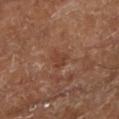| feature | finding |
|---|---|
| tile lighting | cross-polarized |
| patient | aged around 65 |
| location | the left lower leg |
| diameter | ~2.5 mm (longest diameter) |
| TBP lesion metrics | a footprint of about 3.5 mm², an outline eccentricity of about 0.7 (0 = round, 1 = elongated), and a symmetry-axis asymmetry near 0.3; a mean CIELAB color near L≈39 a*≈22 b*≈29 and roughly 6 lightness units darker than nearby skin; a border-irregularity rating of about 3/10, a within-lesion color-variation index near 1.5/10, and radial color variation of about 0.5; a classifier nevus-likeness of about 0/100 and a lesion-detection confidence of about 100/100 |
| imaging modality | total-body-photography crop, ~15 mm field of view |